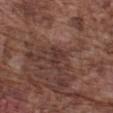This lesion was catalogued during total-body skin photography and was not selected for biopsy. Imaged with white-light lighting. A 15 mm crop from a total-body photograph taken for skin-cancer surveillance. A male patient aged approximately 75. The lesion's longest dimension is about 3 mm. From the left forearm.